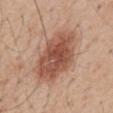Findings:
• notes: imaged on a skin check; not biopsied
• image source: ~15 mm tile from a whole-body skin photo
• anatomic site: the mid back
• lesion diameter: ≈7 mm
• illumination: white-light illumination
• subject: male, in their mid- to late 50s